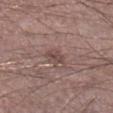The subject is a male aged around 45. Captured under white-light illumination. A lesion tile, about 15 mm wide, cut from a 3D total-body photograph. The total-body-photography lesion software estimated a lesion area of about 2.5 mm² and a symmetry-axis asymmetry near 0.35. It also reported a border-irregularity index near 3.5/10, a within-lesion color-variation index near 0/10, and a peripheral color-asymmetry measure near 0. The analysis additionally found a classifier nevus-likeness of about 0/100. Located on the left lower leg.